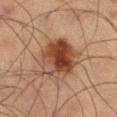Clinical impression:
This lesion was catalogued during total-body skin photography and was not selected for biopsy.